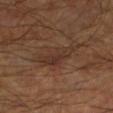Background:
This is a cross-polarized tile. About 5 mm across. The lesion is on the left lower leg. The lesion-visualizer software estimated a mean CIELAB color near L≈32 a*≈17 b*≈24, a lesion–skin lightness drop of about 6, and a normalized border contrast of about 6. A male patient roughly 60 years of age. A lesion tile, about 15 mm wide, cut from a 3D total-body photograph.